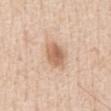Imaged during a routine full-body skin examination; the lesion was not biopsied and no histopathology is available.
Longest diameter approximately 3 mm.
Captured under white-light illumination.
On the front of the torso.
An algorithmic analysis of the crop reported a lesion area of about 7 mm², an outline eccentricity of about 0.5 (0 = round, 1 = elongated), and two-axis asymmetry of about 0.25. It also reported a border-irregularity rating of about 2/10, a color-variation rating of about 4/10, and a peripheral color-asymmetry measure near 1.5.
A lesion tile, about 15 mm wide, cut from a 3D total-body photograph.
A male subject approximately 60 years of age.